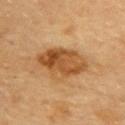Q: Was a biopsy performed?
A: imaged on a skin check; not biopsied
Q: Patient demographics?
A: female, aged 58 to 62
Q: Illumination type?
A: cross-polarized illumination
Q: What is the lesion's diameter?
A: ≈6.5 mm
Q: Automated lesion metrics?
A: a lesion area of about 18 mm²; a lesion color around L≈45 a*≈20 b*≈37 in CIELAB, a lesion–skin lightness drop of about 11, and a lesion-to-skin contrast of about 9 (normalized; higher = more distinct); border irregularity of about 2 on a 0–10 scale, a within-lesion color-variation index near 6.5/10, and peripheral color asymmetry of about 2.5
Q: Where on the body is the lesion?
A: the upper back
Q: How was this image acquired?
A: ~15 mm tile from a whole-body skin photo